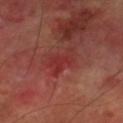Case summary:
- notes: no biopsy performed (imaged during a skin exam)
- lesion size: ~4.5 mm (longest diameter)
- patient: male, aged 68 to 72
- imaging modality: total-body-photography crop, ~15 mm field of view
- lighting: cross-polarized
- TBP lesion metrics: a shape eccentricity near 0.85 and a shape-asymmetry score of about 0.5 (0 = symmetric); an average lesion color of about L≈33 a*≈32 b*≈25 (CIELAB), about 6 CIELAB-L* units darker than the surrounding skin, and a normalized border contrast of about 6; a classifier nevus-likeness of about 0/100 and a lesion-detection confidence of about 85/100
- site: the right lower leg